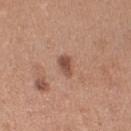Clinical impression:
Recorded during total-body skin imaging; not selected for excision or biopsy.
Context:
A female patient, roughly 30 years of age. Approximately 2.5 mm at its widest. A close-up tile cropped from a whole-body skin photograph, about 15 mm across. From the left upper arm. The total-body-photography lesion software estimated an area of roughly 3.5 mm², an outline eccentricity of about 0.8 (0 = round, 1 = elongated), and two-axis asymmetry of about 0.35.